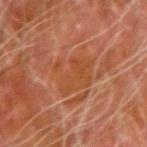Clinical impression: No biopsy was performed on this lesion — it was imaged during a full skin examination and was not determined to be concerning. Context: A 15 mm close-up extracted from a 3D total-body photography capture. The lesion is located on the arm. Automated image analysis of the tile measured a mean CIELAB color near L≈37 a*≈22 b*≈30, a lesion–skin lightness drop of about 4, and a normalized lesion–skin contrast near 5.5. The analysis additionally found a border-irregularity rating of about 5.5/10 and a color-variation rating of about 3/10. The subject is a male aged 78–82. The recorded lesion diameter is about 5.5 mm. This is a cross-polarized tile.